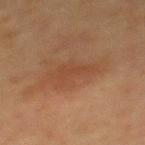{
  "biopsy_status": "not biopsied; imaged during a skin examination",
  "image": {
    "source": "total-body photography crop",
    "field_of_view_mm": 15
  },
  "lighting": "cross-polarized",
  "site": "upper back",
  "lesion_size": {
    "long_diameter_mm_approx": 4.0
  },
  "patient": {
    "sex": "female",
    "age_approx": 50
  },
  "automated_metrics": {
    "area_mm2_approx": 6.5,
    "shape_asymmetry": 0.45,
    "border_irregularity_0_10": 5.5,
    "color_variation_0_10": 1.0,
    "peripheral_color_asymmetry": 0.5,
    "nevus_likeness_0_100": 15
  }
}